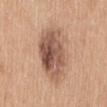Impression: The lesion was tiled from a total-body skin photograph and was not biopsied. Clinical summary: A close-up tile cropped from a whole-body skin photograph, about 15 mm across. Captured under white-light illumination. The patient is a female aged around 55. The lesion is on the mid back. About 7 mm across.